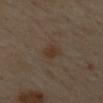Notes:
• notes — imaged on a skin check; not biopsied
• illumination — cross-polarized illumination
• imaging modality — 15 mm crop, total-body photography
• image-analysis metrics — an average lesion color of about L≈33 a*≈13 b*≈24 (CIELAB), about 6 CIELAB-L* units darker than the surrounding skin, and a lesion-to-skin contrast of about 7 (normalized; higher = more distinct); a color-variation rating of about 1.5/10 and radial color variation of about 0.5
• site — the mid back
• subject — male, aged 63–67